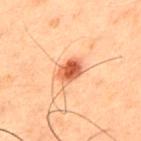Assessment: Recorded during total-body skin imaging; not selected for excision or biopsy. Context: A male subject, in their 50s. A 15 mm close-up extracted from a 3D total-body photography capture. The lesion is on the chest.